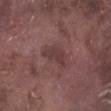follow-up: catalogued during a skin exam; not biopsied | lesion size: about 3.5 mm | automated lesion analysis: a lesion-detection confidence of about 70/100 | tile lighting: white-light illumination | acquisition: ~15 mm crop, total-body skin-cancer survey | patient: male, in their mid-70s | anatomic site: the right lower leg.